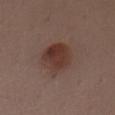{"biopsy_status": "not biopsied; imaged during a skin examination", "lesion_size": {"long_diameter_mm_approx": 4.0}, "patient": {"sex": "male", "age_approx": 40}, "automated_metrics": {"cielab_L": 36, "cielab_a": 19, "cielab_b": 23, "vs_skin_darker_L": 9.0, "vs_skin_contrast_norm": 8.5, "border_irregularity_0_10": 2.0, "color_variation_0_10": 4.5, "peripheral_color_asymmetry": 1.5}, "site": "chest", "image": {"source": "total-body photography crop", "field_of_view_mm": 15}}